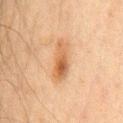<case>
  <image>
    <source>total-body photography crop</source>
    <field_of_view_mm>15</field_of_view_mm>
  </image>
  <patient>
    <sex>male</sex>
    <age_approx>60</age_approx>
  </patient>
  <site>chest</site>
</case>